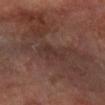Assessment:
Part of a total-body skin-imaging series; this lesion was reviewed on a skin check and was not flagged for biopsy.
Clinical summary:
Located on the left arm. A female subject aged 63–67. Cropped from a whole-body photographic skin survey; the tile spans about 15 mm.